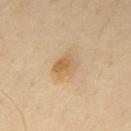Imaged during a routine full-body skin examination; the lesion was not biopsied and no histopathology is available. The lesion is on the back. A male patient, aged approximately 50. Cropped from a total-body skin-imaging series; the visible field is about 15 mm.